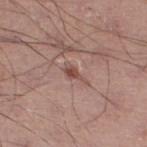| field | value |
|---|---|
| workup | no biopsy performed (imaged during a skin exam) |
| imaging modality | 15 mm crop, total-body photography |
| lesion size | ≈3 mm |
| illumination | white-light illumination |
| patient | male, aged around 50 |
| automated metrics | a lesion color around L≈49 a*≈21 b*≈24 in CIELAB and a normalized lesion–skin contrast near 7 |
| site | the leg |